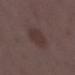{"biopsy_status": "not biopsied; imaged during a skin examination", "site": "right lower leg", "lighting": "white-light", "patient": {"sex": "female", "age_approx": 35}, "image": {"source": "total-body photography crop", "field_of_view_mm": 15}, "automated_metrics": {"area_mm2_approx": 9.0, "eccentricity": 0.65, "shape_asymmetry": 0.15}}